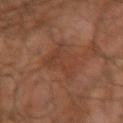Recorded during total-body skin imaging; not selected for excision or biopsy.
A male subject, in their mid-60s.
The lesion's longest dimension is about 6 mm.
An algorithmic analysis of the crop reported a lesion color around L≈37 a*≈21 b*≈28 in CIELAB, roughly 6 lightness units darker than nearby skin, and a normalized border contrast of about 5. And it measured a border-irregularity rating of about 5.5/10, internal color variation of about 3 on a 0–10 scale, and a peripheral color-asymmetry measure near 1.
The tile uses cross-polarized illumination.
The lesion is on the right upper arm.
Cropped from a whole-body photographic skin survey; the tile spans about 15 mm.